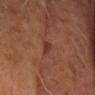Clinical impression: Part of a total-body skin-imaging series; this lesion was reviewed on a skin check and was not flagged for biopsy. Image and clinical context: A male subject, in their 70s. Imaged with cross-polarized lighting. A 15 mm close-up extracted from a 3D total-body photography capture. The lesion's longest dimension is about 3 mm. An algorithmic analysis of the crop reported a footprint of about 2.5 mm² and a shape-asymmetry score of about 0.35 (0 = symmetric). The software also gave roughly 8 lightness units darker than nearby skin. The analysis additionally found a within-lesion color-variation index near 0/10 and radial color variation of about 0. From the head or neck.